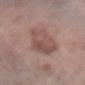workup: total-body-photography surveillance lesion; no biopsy | patient: female, roughly 70 years of age | image source: ~15 mm crop, total-body skin-cancer survey | location: the arm.